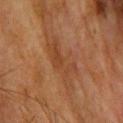Impression: This lesion was catalogued during total-body skin photography and was not selected for biopsy. Image and clinical context: A female subject, roughly 55 years of age. A 15 mm close-up tile from a total-body photography series done for melanoma screening. About 6.5 mm across. The lesion is located on the upper back.